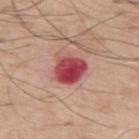{
  "biopsy_status": "not biopsied; imaged during a skin examination",
  "patient": {
    "sex": "male",
    "age_approx": 70
  },
  "image": {
    "source": "total-body photography crop",
    "field_of_view_mm": 15
  },
  "site": "upper back",
  "automated_metrics": {
    "area_mm2_approx": 9.0,
    "eccentricity": 0.6,
    "shape_asymmetry": 0.2,
    "border_irregularity_0_10": 2.0,
    "color_variation_0_10": 5.5,
    "peripheral_color_asymmetry": 1.5
  },
  "lighting": "white-light",
  "lesion_size": {
    "long_diameter_mm_approx": 3.5
  }
}